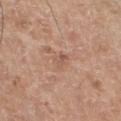Case summary:
* workup: imaged on a skin check; not biopsied
* subject: male, aged approximately 60
* TBP lesion metrics: a footprint of about 3 mm², a shape eccentricity near 0.8, and two-axis asymmetry of about 0.45; a mean CIELAB color near L≈56 a*≈21 b*≈29, a lesion–skin lightness drop of about 7, and a lesion-to-skin contrast of about 5 (normalized; higher = more distinct); a lesion-detection confidence of about 100/100
* acquisition: ~15 mm crop, total-body skin-cancer survey
* site: the left lower leg
* lesion size: ≈2.5 mm
* lighting: white-light illumination